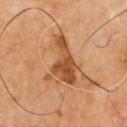Captured during whole-body skin photography for melanoma surveillance; the lesion was not biopsied. Imaged with cross-polarized lighting. The lesion's longest dimension is about 6 mm. On the chest. A 15 mm close-up extracted from a 3D total-body photography capture.